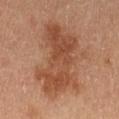Part of a total-body skin-imaging series; this lesion was reviewed on a skin check and was not flagged for biopsy. The patient is a female approximately 60 years of age. From the left thigh. Approximately 9.5 mm at its widest. A roughly 15 mm field-of-view crop from a total-body skin photograph. Captured under cross-polarized illumination.